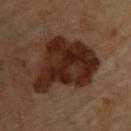Part of a total-body skin-imaging series; this lesion was reviewed on a skin check and was not flagged for biopsy. The lesion is on the upper back. Longest diameter approximately 9 mm. Captured under cross-polarized illumination. A 15 mm close-up extracted from a 3D total-body photography capture. A female subject aged 68–72. Automated tile analysis of the lesion measured a lesion area of about 34 mm², an outline eccentricity of about 0.75 (0 = round, 1 = elongated), and a symmetry-axis asymmetry near 0.35. The software also gave roughly 13 lightness units darker than nearby skin and a lesion-to-skin contrast of about 13.5 (normalized; higher = more distinct).